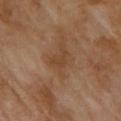{
  "image": {
    "source": "total-body photography crop",
    "field_of_view_mm": 15
  },
  "patient": {
    "sex": "male",
    "age_approx": 70
  },
  "site": "upper back",
  "lesion_size": {
    "long_diameter_mm_approx": 3.0
  }
}